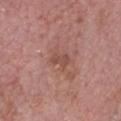Clinical impression: Captured during whole-body skin photography for melanoma surveillance; the lesion was not biopsied. Image and clinical context: This is a white-light tile. The recorded lesion diameter is about 2.5 mm. Located on the head or neck. An algorithmic analysis of the crop reported roughly 8 lightness units darker than nearby skin. It also reported radial color variation of about 0. It also reported a detector confidence of about 100 out of 100 that the crop contains a lesion. This image is a 15 mm lesion crop taken from a total-body photograph. A male subject, in their 60s.